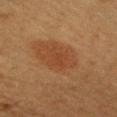Clinical impression:
Part of a total-body skin-imaging series; this lesion was reviewed on a skin check and was not flagged for biopsy.
Context:
On the chest. The subject is a male aged around 40. Imaged with cross-polarized lighting. Automated image analysis of the tile measured an automated nevus-likeness rating near 100 out of 100 and a detector confidence of about 100 out of 100 that the crop contains a lesion. A 15 mm close-up tile from a total-body photography series done for melanoma screening.